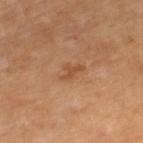The lesion was tiled from a total-body skin photograph and was not biopsied.
A close-up tile cropped from a whole-body skin photograph, about 15 mm across.
The lesion is on the right lower leg.
The lesion-visualizer software estimated a lesion area of about 3.5 mm², an outline eccentricity of about 0.8 (0 = round, 1 = elongated), and a symmetry-axis asymmetry near 0.6. And it measured a lesion color around L≈51 a*≈22 b*≈36 in CIELAB, a lesion–skin lightness drop of about 7, and a normalized border contrast of about 5.5. And it measured border irregularity of about 5.5 on a 0–10 scale, a within-lesion color-variation index near 0/10, and peripheral color asymmetry of about 0.
Captured under cross-polarized illumination.
The recorded lesion diameter is about 3 mm.
A male subject, aged 63–67.